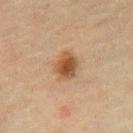Recorded during total-body skin imaging; not selected for excision or biopsy. The recorded lesion diameter is about 3.5 mm. A region of skin cropped from a whole-body photographic capture, roughly 15 mm wide. From the abdomen. A male patient aged 68–72. The tile uses cross-polarized illumination.